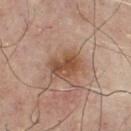On the chest.
A male subject aged 78 to 82.
This image is a 15 mm lesion crop taken from a total-body photograph.
The tile uses white-light illumination.
The lesion's longest dimension is about 4 mm.